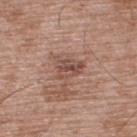This lesion was catalogued during total-body skin photography and was not selected for biopsy. A lesion tile, about 15 mm wide, cut from a 3D total-body photograph. The lesion-visualizer software estimated an average lesion color of about L≈49 a*≈20 b*≈25 (CIELAB), about 9 CIELAB-L* units darker than the surrounding skin, and a normalized border contrast of about 6.5. The software also gave a border-irregularity rating of about 3/10. The analysis additionally found an automated nevus-likeness rating near 0 out of 100. A male patient aged around 50. The tile uses white-light illumination. The lesion's longest dimension is about 3.5 mm. Located on the upper back.